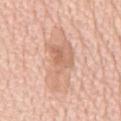Case summary:
* biopsy status · imaged on a skin check; not biopsied
* tile lighting · white-light illumination
* body site · the chest
* patient · male, aged 73–77
* size · ≈7.5 mm
* imaging modality · ~15 mm tile from a whole-body skin photo